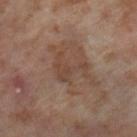Assessment: Imaged during a routine full-body skin examination; the lesion was not biopsied and no histopathology is available. Image and clinical context: Imaged with cross-polarized lighting. Cropped from a whole-body photographic skin survey; the tile spans about 15 mm. On the right thigh. The patient is a female aged 53–57. About 4 mm across. The lesion-visualizer software estimated border irregularity of about 10 on a 0–10 scale, internal color variation of about 1 on a 0–10 scale, and a peripheral color-asymmetry measure near 0.5.